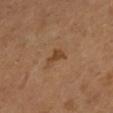  biopsy_status: not biopsied; imaged during a skin examination
  image:
    source: total-body photography crop
    field_of_view_mm: 15
  lighting: cross-polarized
  automated_metrics:
    area_mm2_approx: 3.0
    shape_asymmetry: 0.45
  site: leg
  patient:
    sex: female
    age_approx: 30